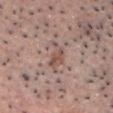Recorded during total-body skin imaging; not selected for excision or biopsy. A male subject, aged 48–52. Captured under white-light illumination. Approximately 4.5 mm at its widest. Located on the head or neck. A 15 mm close-up tile from a total-body photography series done for melanoma screening.